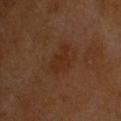The lesion was tiled from a total-body skin photograph and was not biopsied. This image is a 15 mm lesion crop taken from a total-body photograph. The tile uses cross-polarized illumination. A male patient, approximately 60 years of age. Approximately 3.5 mm at its widest. From the head or neck.